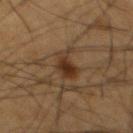Clinical impression: Part of a total-body skin-imaging series; this lesion was reviewed on a skin check and was not flagged for biopsy. Background: A male patient, in their mid- to late 50s. This image is a 15 mm lesion crop taken from a total-body photograph. Located on the chest.